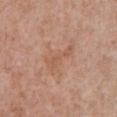The lesion was photographed on a routine skin check and not biopsied; there is no pathology result.
A 15 mm crop from a total-body photograph taken for skin-cancer surveillance.
The recorded lesion diameter is about 4.5 mm.
A male patient about 70 years old.
The tile uses white-light illumination.
The lesion is located on the chest.